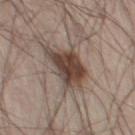workup = total-body-photography surveillance lesion; no biopsy
patient = male, aged around 55
site = the leg
image source = 15 mm crop, total-body photography
diameter = about 5 mm